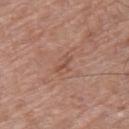Imaged during a routine full-body skin examination; the lesion was not biopsied and no histopathology is available.
The lesion is located on the right thigh.
Cropped from a whole-body photographic skin survey; the tile spans about 15 mm.
Imaged with white-light lighting.
A male patient, aged approximately 60.
The lesion's longest dimension is about 2.5 mm.